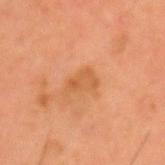Q: Was a biopsy performed?
A: catalogued during a skin exam; not biopsied
Q: What did automated image analysis measure?
A: a lesion area of about 5 mm², an eccentricity of roughly 0.8, and a shape-asymmetry score of about 0.35 (0 = symmetric); border irregularity of about 3.5 on a 0–10 scale, internal color variation of about 3 on a 0–10 scale, and a peripheral color-asymmetry measure near 1
Q: Who is the patient?
A: male, in their mid- to late 50s
Q: How was this image acquired?
A: ~15 mm crop, total-body skin-cancer survey
Q: How was the tile lit?
A: cross-polarized illumination
Q: How large is the lesion?
A: ≈3.5 mm
Q: Lesion location?
A: the head or neck